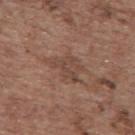notes: imaged on a skin check; not biopsied | site: the upper back | patient: female, in their mid- to late 60s | diameter: ≈4.5 mm | tile lighting: white-light | acquisition: total-body-photography crop, ~15 mm field of view | automated lesion analysis: a mean CIELAB color near L≈45 a*≈18 b*≈26, a lesion–skin lightness drop of about 7, and a lesion-to-skin contrast of about 5.5 (normalized; higher = more distinct); border irregularity of about 5.5 on a 0–10 scale and internal color variation of about 3 on a 0–10 scale; an automated nevus-likeness rating near 0 out of 100.